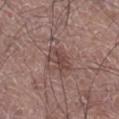Impression: Recorded during total-body skin imaging; not selected for excision or biopsy. Image and clinical context: Captured under white-light illumination. From the leg. The subject is a male roughly 75 years of age. The lesion's longest dimension is about 3.5 mm. Cropped from a total-body skin-imaging series; the visible field is about 15 mm.